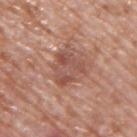No biopsy was performed on this lesion — it was imaged during a full skin examination and was not determined to be concerning. This is a white-light tile. From the right upper arm. A 15 mm crop from a total-body photograph taken for skin-cancer surveillance. The subject is a male roughly 70 years of age.